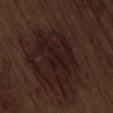notes: imaged on a skin check; not biopsied
acquisition: ~15 mm tile from a whole-body skin photo
patient: male, aged 68–72
site: the lower back